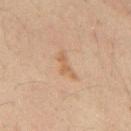Assessment: No biopsy was performed on this lesion — it was imaged during a full skin examination and was not determined to be concerning. Clinical summary: The lesion is on the mid back. Automated tile analysis of the lesion measured an area of roughly 4 mm² and an outline eccentricity of about 0.9 (0 = round, 1 = elongated). The analysis additionally found a border-irregularity index near 5/10 and a color-variation rating of about 0.5/10. The analysis additionally found a classifier nevus-likeness of about 0/100 and a lesion-detection confidence of about 100/100. This is a cross-polarized tile. A 15 mm close-up extracted from a 3D total-body photography capture. A male patient, in their 50s.